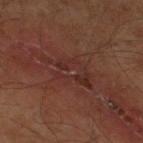The lesion was tiled from a total-body skin photograph and was not biopsied. The tile uses cross-polarized illumination. The subject is a male about 60 years old. A 15 mm crop from a total-body photograph taken for skin-cancer surveillance. The recorded lesion diameter is about 6 mm. The lesion is on the leg.